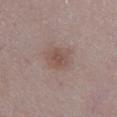The lesion was tiled from a total-body skin photograph and was not biopsied. This is a white-light tile. An algorithmic analysis of the crop reported a mean CIELAB color near L≈50 a*≈16 b*≈23, a lesion–skin lightness drop of about 7, and a normalized lesion–skin contrast near 6. And it measured a border-irregularity rating of about 2.5/10, internal color variation of about 3 on a 0–10 scale, and peripheral color asymmetry of about 1. The analysis additionally found a classifier nevus-likeness of about 40/100 and lesion-presence confidence of about 100/100. Longest diameter approximately 3.5 mm. On the left lower leg. A male subject aged around 80. A close-up tile cropped from a whole-body skin photograph, about 15 mm across.